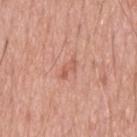Assessment: Imaged during a routine full-body skin examination; the lesion was not biopsied and no histopathology is available. Clinical summary: The lesion-visualizer software estimated a lesion area of about 3 mm². The software also gave a normalized lesion–skin contrast near 5.5. The software also gave a border-irregularity index near 4/10 and peripheral color asymmetry of about 0. And it measured a classifier nevus-likeness of about 0/100 and a detector confidence of about 100 out of 100 that the crop contains a lesion. A male subject aged 68 to 72. Cropped from a whole-body photographic skin survey; the tile spans about 15 mm. From the upper back. This is a white-light tile. The recorded lesion diameter is about 3 mm.